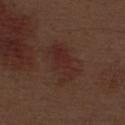Impression:
The lesion was photographed on a routine skin check and not biopsied; there is no pathology result.
Image and clinical context:
A roughly 15 mm field-of-view crop from a total-body skin photograph. From the abdomen. The lesion's longest dimension is about 5.5 mm. A male patient, in their 70s. Captured under white-light illumination. Automated tile analysis of the lesion measured an outline eccentricity of about 0.85 (0 = round, 1 = elongated) and two-axis asymmetry of about 0.25.